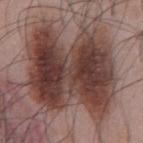No biopsy was performed on this lesion — it was imaged during a full skin examination and was not determined to be concerning.
A male subject, aged 53 to 57.
The lesion-visualizer software estimated an outline eccentricity of about 0.6 (0 = round, 1 = elongated) and a symmetry-axis asymmetry near 0.2. It also reported border irregularity of about 4 on a 0–10 scale, internal color variation of about 8.5 on a 0–10 scale, and radial color variation of about 3.
From the abdomen.
A lesion tile, about 15 mm wide, cut from a 3D total-body photograph.
About 10 mm across.